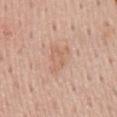Captured during whole-body skin photography for melanoma surveillance; the lesion was not biopsied. The lesion-visualizer software estimated a lesion color around L≈63 a*≈20 b*≈30 in CIELAB, roughly 6 lightness units darker than nearby skin, and a normalized border contrast of about 4.5. The software also gave border irregularity of about 6 on a 0–10 scale and a within-lesion color-variation index near 2/10. A close-up tile cropped from a whole-body skin photograph, about 15 mm across. The recorded lesion diameter is about 4 mm. The lesion is on the mid back. The tile uses white-light illumination. The patient is a male approximately 55 years of age.